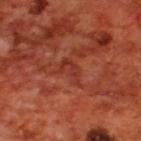Automated image analysis of the tile measured a lesion color around L≈35 a*≈31 b*≈31 in CIELAB, a lesion–skin lightness drop of about 6, and a normalized lesion–skin contrast near 5.5. And it measured border irregularity of about 7 on a 0–10 scale. And it measured an automated nevus-likeness rating near 0 out of 100 and lesion-presence confidence of about 75/100.
A close-up tile cropped from a whole-body skin photograph, about 15 mm across.
Imaged with cross-polarized lighting.
Measured at roughly 3 mm in maximum diameter.
A male subject about 70 years old.
On the upper back.